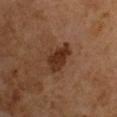follow-up: total-body-photography surveillance lesion; no biopsy | lesion diameter: ~4 mm (longest diameter) | acquisition: ~15 mm tile from a whole-body skin photo | illumination: cross-polarized | subject: male, in their mid-60s.